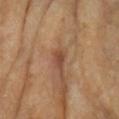Imaged with cross-polarized lighting.
The patient is a female aged around 75.
Automated image analysis of the tile measured an area of roughly 3 mm², a shape eccentricity near 0.85, and two-axis asymmetry of about 0.3. The software also gave a lesion color around L≈46 a*≈22 b*≈31 in CIELAB, roughly 9 lightness units darker than nearby skin, and a normalized border contrast of about 7.
A 15 mm close-up extracted from a 3D total-body photography capture.
Approximately 2.5 mm at its widest.
The lesion is on the left forearm.